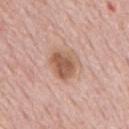Q: Was a biopsy performed?
A: total-body-photography surveillance lesion; no biopsy
Q: Automated lesion metrics?
A: a lesion area of about 10 mm²; an average lesion color of about L≈58 a*≈20 b*≈30 (CIELAB), a lesion–skin lightness drop of about 12, and a normalized lesion–skin contrast near 8; border irregularity of about 2.5 on a 0–10 scale, a color-variation rating of about 5/10, and peripheral color asymmetry of about 1.5
Q: Illumination type?
A: white-light
Q: What kind of image is this?
A: ~15 mm crop, total-body skin-cancer survey
Q: Patient demographics?
A: male, aged 73–77
Q: Where on the body is the lesion?
A: the mid back